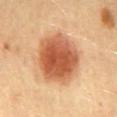Q: Was a biopsy performed?
A: total-body-photography surveillance lesion; no biopsy
Q: What did automated image analysis measure?
A: border irregularity of about 1 on a 0–10 scale and a within-lesion color-variation index near 6/10; a classifier nevus-likeness of about 100/100 and lesion-presence confidence of about 100/100
Q: What are the patient's age and sex?
A: female, aged 58–62
Q: Where on the body is the lesion?
A: the lower back
Q: What kind of image is this?
A: total-body-photography crop, ~15 mm field of view